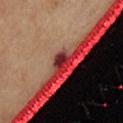Findings:
– notes — no biopsy performed (imaged during a skin exam)
– body site — the chest
– diameter — ~2.5 mm (longest diameter)
– image source — ~15 mm crop, total-body skin-cancer survey
– illumination — cross-polarized
– subject — female, aged 48 to 52
– image-analysis metrics — a footprint of about 4 mm² and an outline eccentricity of about 0.7 (0 = round, 1 = elongated); internal color variation of about 4 on a 0–10 scale and radial color variation of about 1.5